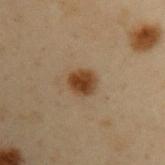workup: imaged on a skin check; not biopsied | tile lighting: cross-polarized | TBP lesion metrics: a lesion area of about 6 mm² and a symmetry-axis asymmetry near 0.15; a border-irregularity rating of about 1.5/10, a within-lesion color-variation index near 3/10, and a peripheral color-asymmetry measure near 1; a lesion-detection confidence of about 100/100 | diameter: about 3 mm | image: ~15 mm tile from a whole-body skin photo | location: the left upper arm | subject: male, in their mid- to late 50s.